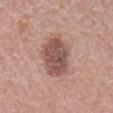The lesion was photographed on a routine skin check and not biopsied; there is no pathology result. The patient is a male aged around 70. Located on the mid back. Cropped from a total-body skin-imaging series; the visible field is about 15 mm.